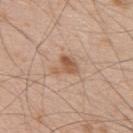Impression: Part of a total-body skin-imaging series; this lesion was reviewed on a skin check and was not flagged for biopsy. Context: The tile uses white-light illumination. Cropped from a total-body skin-imaging series; the visible field is about 15 mm. Approximately 3 mm at its widest. The total-body-photography lesion software estimated an eccentricity of roughly 0.65 and a symmetry-axis asymmetry near 0.5. Located on the back. A male subject aged 48–52.